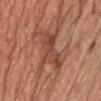Captured during whole-body skin photography for melanoma surveillance; the lesion was not biopsied. Cropped from a total-body skin-imaging series; the visible field is about 15 mm. Imaged with white-light lighting. The patient is a male approximately 60 years of age. The lesion is on the chest.